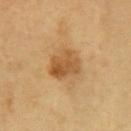{"biopsy_status": "not biopsied; imaged during a skin examination", "patient": {"sex": "male", "age_approx": 65}, "automated_metrics": {"area_mm2_approx": 10.0, "eccentricity": 0.65, "border_irregularity_0_10": 2.0, "color_variation_0_10": 5.0, "peripheral_color_asymmetry": 2.0, "lesion_detection_confidence_0_100": 100}, "image": {"source": "total-body photography crop", "field_of_view_mm": 15}, "lighting": "cross-polarized", "site": "right upper arm"}